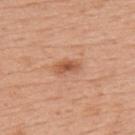notes: no biopsy performed (imaged during a skin exam)
image source: ~15 mm crop, total-body skin-cancer survey
image-analysis metrics: an eccentricity of roughly 0.85 and a symmetry-axis asymmetry near 0.25
lighting: white-light illumination
subject: female, about 50 years old
body site: the back